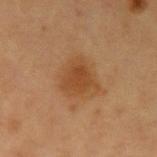biopsy status=imaged on a skin check; not biopsied | illumination=cross-polarized illumination | lesion diameter=about 4 mm | body site=the left upper arm | patient=female, in their 40s | image source=15 mm crop, total-body photography.